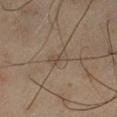follow-up: total-body-photography surveillance lesion; no biopsy | image source: ~15 mm tile from a whole-body skin photo | TBP lesion metrics: a lesion area of about 3 mm², an outline eccentricity of about 0.9 (0 = round, 1 = elongated), and a symmetry-axis asymmetry near 0.4; roughly 5 lightness units darker than nearby skin and a normalized border contrast of about 5; an automated nevus-likeness rating near 0 out of 100 and lesion-presence confidence of about 70/100 | tile lighting: cross-polarized | patient: male, aged 43 to 47 | location: the left thigh.